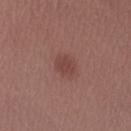No biopsy was performed on this lesion — it was imaged during a full skin examination and was not determined to be concerning.
A 15 mm close-up tile from a total-body photography series done for melanoma screening.
The lesion is on the arm.
The patient is a female in their mid-20s.